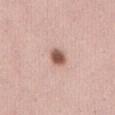Case summary:
– follow-up — catalogued during a skin exam; not biopsied
– subject — female, roughly 45 years of age
– image — total-body-photography crop, ~15 mm field of view
– lesion size — ≈2 mm
– anatomic site — the abdomen